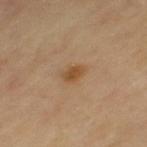follow-up: imaged on a skin check; not biopsied | image-analysis metrics: an area of roughly 3 mm², an outline eccentricity of about 0.8 (0 = round, 1 = elongated), and a symmetry-axis asymmetry near 0.25 | subject: female, approximately 70 years of age | imaging modality: total-body-photography crop, ~15 mm field of view | site: the leg | illumination: cross-polarized | lesion diameter: about 2.5 mm.